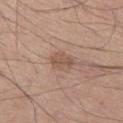On the left thigh. About 2.5 mm across. A close-up tile cropped from a whole-body skin photograph, about 15 mm across. The subject is a male approximately 60 years of age.